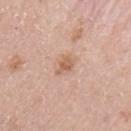<tbp_lesion>
<lesion_size>
  <long_diameter_mm_approx>2.5</long_diameter_mm_approx>
</lesion_size>
<automated_metrics>
  <area_mm2_approx>4.0</area_mm2_approx>
  <eccentricity>0.7</eccentricity>
  <shape_asymmetry>0.35</shape_asymmetry>
  <cielab_L>61</cielab_L>
  <cielab_a>20</cielab_a>
  <cielab_b>31</cielab_b>
  <vs_skin_darker_L>9.0</vs_skin_darker_L>
  <border_irregularity_0_10>3.5</border_irregularity_0_10>
  <color_variation_0_10>2.0</color_variation_0_10>
  <nevus_likeness_0_100>10</nevus_likeness_0_100>
</automated_metrics>
<lighting>white-light</lighting>
<image>
  <source>total-body photography crop</source>
  <field_of_view_mm>15</field_of_view_mm>
</image>
<patient>
  <sex>female</sex>
  <age_approx>50</age_approx>
</patient>
<site>left upper arm</site>
</tbp_lesion>